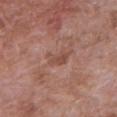Impression: The lesion was photographed on a routine skin check and not biopsied; there is no pathology result. Acquisition and patient details: The tile uses white-light illumination. A female subject aged 68–72. Automated tile analysis of the lesion measured a nevus-likeness score of about 0/100 and a detector confidence of about 100 out of 100 that the crop contains a lesion. The lesion's longest dimension is about 2.5 mm. A lesion tile, about 15 mm wide, cut from a 3D total-body photograph. Located on the right upper arm.